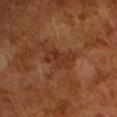{"biopsy_status": "not biopsied; imaged during a skin examination", "lesion_size": {"long_diameter_mm_approx": 4.5}, "image": {"source": "total-body photography crop", "field_of_view_mm": 15}, "patient": {"sex": "male", "age_approx": 65}, "automated_metrics": {"area_mm2_approx": 7.0, "eccentricity": 0.9, "shape_asymmetry": 0.35, "cielab_L": 33, "cielab_a": 23, "cielab_b": 31, "vs_skin_darker_L": 7.0}}